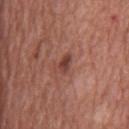biopsy status: total-body-photography surveillance lesion; no biopsy | subject: male, aged approximately 75 | imaging modality: ~15 mm crop, total-body skin-cancer survey | body site: the chest | tile lighting: white-light.